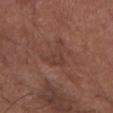workup = total-body-photography surveillance lesion; no biopsy
imaging modality = total-body-photography crop, ~15 mm field of view
tile lighting = white-light illumination
subject = male, aged approximately 75
location = the right forearm